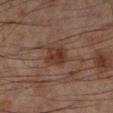This lesion was catalogued during total-body skin photography and was not selected for biopsy.